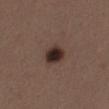{"biopsy_status": "not biopsied; imaged during a skin examination", "automated_metrics": {"area_mm2_approx": 6.5, "eccentricity": 0.5, "shape_asymmetry": 0.2, "cielab_L": 29, "cielab_a": 15, "cielab_b": 20, "vs_skin_darker_L": 14.0}, "image": {"source": "total-body photography crop", "field_of_view_mm": 15}, "site": "left thigh", "lighting": "white-light", "patient": {"sex": "female", "age_approx": 40}}